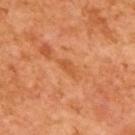workup — catalogued during a skin exam; not biopsied
subject — male, aged 63–67
image — ~15 mm crop, total-body skin-cancer survey
illumination — cross-polarized illumination